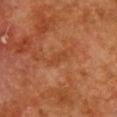Findings:
* workup — catalogued during a skin exam; not biopsied
* subject — female, about 50 years old
* image source — 15 mm crop, total-body photography
* automated metrics — an average lesion color of about L≈36 a*≈22 b*≈31 (CIELAB), about 5 CIELAB-L* units darker than the surrounding skin, and a lesion-to-skin contrast of about 4.5 (normalized; higher = more distinct); a border-irregularity rating of about 4/10, a within-lesion color-variation index near 1/10, and radial color variation of about 0; an automated nevus-likeness rating near 0 out of 100 and lesion-presence confidence of about 100/100
* illumination — cross-polarized illumination
* site — the front of the torso
* lesion diameter — ~3 mm (longest diameter)